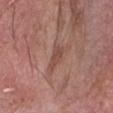No biopsy was performed on this lesion — it was imaged during a full skin examination and was not determined to be concerning. A male subject aged approximately 65. A 15 mm crop from a total-body photograph taken for skin-cancer surveillance. The lesion is located on the head or neck.